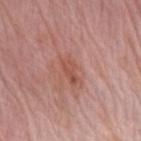automated_metrics:
  area_mm2_approx: 4.5
  eccentricity: 0.9
  border_irregularity_0_10: 3.5
  color_variation_0_10: 1.5
  peripheral_color_asymmetry: 0.5
  nevus_likeness_0_100: 0
  lesion_detection_confidence_0_100: 100
patient:
  sex: female
  age_approx: 65
lesion_size:
  long_diameter_mm_approx: 3.5
site: left forearm
lighting: white-light
image:
  source: total-body photography crop
  field_of_view_mm: 15